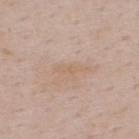| feature | finding |
|---|---|
| biopsy status | total-body-photography surveillance lesion; no biopsy |
| patient | male, in their 50s |
| imaging modality | ~15 mm crop, total-body skin-cancer survey |
| location | the upper back |